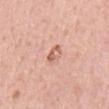Assessment:
Imaged during a routine full-body skin examination; the lesion was not biopsied and no histopathology is available.
Clinical summary:
Cropped from a total-body skin-imaging series; the visible field is about 15 mm. On the chest. Imaged with white-light lighting. About 3 mm across. A male subject, aged approximately 55.